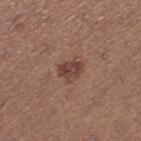A 15 mm close-up tile from a total-body photography series done for melanoma screening.
A female patient, aged approximately 25.
The lesion is located on the leg.
Imaged with white-light lighting.
Measured at roughly 2.5 mm in maximum diameter.